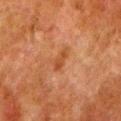Located on the leg. A 15 mm crop from a total-body photograph taken for skin-cancer surveillance. A male patient, about 80 years old.